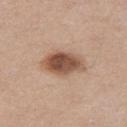Part of a total-body skin-imaging series; this lesion was reviewed on a skin check and was not flagged for biopsy.
Cropped from a total-body skin-imaging series; the visible field is about 15 mm.
Imaged with white-light lighting.
On the left thigh.
The patient is a female aged 38–42.
Longest diameter approximately 5.5 mm.